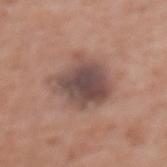Context:
The lesion is located on the back. About 5.5 mm across. An algorithmic analysis of the crop reported a shape eccentricity near 0.5. And it measured an average lesion color of about L≈47 a*≈18 b*≈21 (CIELAB), a lesion–skin lightness drop of about 12, and a lesion-to-skin contrast of about 10 (normalized; higher = more distinct). And it measured border irregularity of about 2.5 on a 0–10 scale, a within-lesion color-variation index near 5.5/10, and a peripheral color-asymmetry measure near 1.5. A female patient roughly 75 years of age. A roughly 15 mm field-of-view crop from a total-body skin photograph.